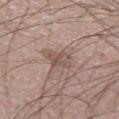Q: What are the patient's age and sex?
A: male, aged around 20
Q: Lesion location?
A: the left thigh
Q: How was this image acquired?
A: ~15 mm tile from a whole-body skin photo
Q: Automated lesion metrics?
A: a footprint of about 5.5 mm² and a shape-asymmetry score of about 0.25 (0 = symmetric); a lesion color around L≈52 a*≈16 b*≈23 in CIELAB, about 8 CIELAB-L* units darker than the surrounding skin, and a normalized border contrast of about 6; a border-irregularity rating of about 3.5/10, a color-variation rating of about 3/10, and radial color variation of about 1
Q: Illumination type?
A: white-light illumination